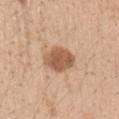Notes:
• size · ~4 mm (longest diameter)
• anatomic site · the left upper arm
• tile lighting · white-light illumination
• patient · female, aged around 25
• image source · 15 mm crop, total-body photography